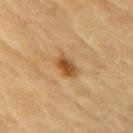Imaged during a routine full-body skin examination; the lesion was not biopsied and no histopathology is available. Measured at roughly 3 mm in maximum diameter. A male patient, aged around 85 or older. The total-body-photography lesion software estimated an area of roughly 4.5 mm² and an eccentricity of roughly 0.75. The analysis additionally found a border-irregularity rating of about 2/10, a color-variation rating of about 5/10, and radial color variation of about 1.5. This image is a 15 mm lesion crop taken from a total-body photograph. Located on the right upper arm.